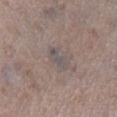Findings:
* location — the leg
* lighting — white-light
* size — about 3 mm
* imaging modality — ~15 mm crop, total-body skin-cancer survey
* subject — female, aged 68 to 72